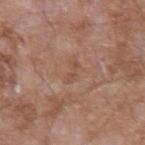Findings:
- follow-up · total-body-photography surveillance lesion; no biopsy
- image source · 15 mm crop, total-body photography
- automated metrics · a footprint of about 2.5 mm², a shape eccentricity near 0.9, and a symmetry-axis asymmetry near 0.45; a lesion color around L≈50 a*≈20 b*≈29 in CIELAB, roughly 6 lightness units darker than nearby skin, and a normalized border contrast of about 5
- lesion size · ≈2.5 mm
- site · the arm
- subject · male, aged around 60
- lighting · white-light